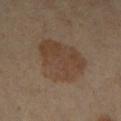notes: imaged on a skin check; not biopsied
lesion diameter: ~6 mm (longest diameter)
patient: aged 58–62
image source: total-body-photography crop, ~15 mm field of view
illumination: cross-polarized
anatomic site: the leg
TBP lesion metrics: roughly 7 lightness units darker than nearby skin and a lesion-to-skin contrast of about 6.5 (normalized; higher = more distinct); internal color variation of about 3.5 on a 0–10 scale; a classifier nevus-likeness of about 30/100